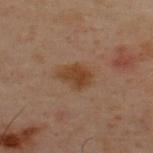Clinical impression: Captured during whole-body skin photography for melanoma surveillance; the lesion was not biopsied. Clinical summary: The recorded lesion diameter is about 4 mm. A male subject, approximately 50 years of age. The lesion is located on the back. A 15 mm close-up extracted from a 3D total-body photography capture.